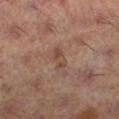notes: imaged on a skin check; not biopsied | site: the right lower leg | tile lighting: cross-polarized | automated lesion analysis: a lesion area of about 4 mm², a shape eccentricity near 0.9, and a shape-asymmetry score of about 0.3 (0 = symmetric); a normalized lesion–skin contrast near 5.5; border irregularity of about 3 on a 0–10 scale, internal color variation of about 5 on a 0–10 scale, and peripheral color asymmetry of about 1.5 | imaging modality: total-body-photography crop, ~15 mm field of view | subject: female, approximately 60 years of age | lesion size: about 3 mm.